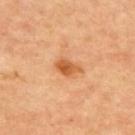Impression:
The lesion was photographed on a routine skin check and not biopsied; there is no pathology result.
Context:
The subject is aged approximately 65. From the upper back. The total-body-photography lesion software estimated an area of roughly 5 mm² and an outline eccentricity of about 0.8 (0 = round, 1 = elongated). The analysis additionally found a lesion color around L≈58 a*≈27 b*≈43 in CIELAB, roughly 12 lightness units darker than nearby skin, and a normalized border contrast of about 8. The software also gave a nevus-likeness score of about 80/100 and a lesion-detection confidence of about 100/100. Cropped from a total-body skin-imaging series; the visible field is about 15 mm. Approximately 3 mm at its widest. Imaged with cross-polarized lighting.